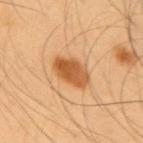Q: Was this lesion biopsied?
A: catalogued during a skin exam; not biopsied
Q: What are the patient's age and sex?
A: male, aged around 55
Q: What kind of image is this?
A: ~15 mm tile from a whole-body skin photo
Q: What is the anatomic site?
A: the upper back